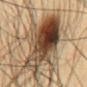- workup: no biopsy performed (imaged during a skin exam)
- site: the mid back
- acquisition: 15 mm crop, total-body photography
- patient: male, aged 33 to 37